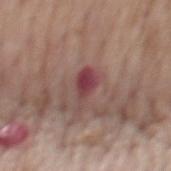Q: Is there a histopathology result?
A: catalogued during a skin exam; not biopsied
Q: What is the lesion's diameter?
A: about 4 mm
Q: How was the tile lit?
A: white-light illumination
Q: What kind of image is this?
A: total-body-photography crop, ~15 mm field of view
Q: What is the anatomic site?
A: the mid back
Q: Who is the patient?
A: male, in their mid- to late 70s